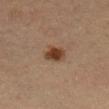Case summary:
• follow-up: catalogued during a skin exam; not biopsied
• tile lighting: cross-polarized illumination
• automated metrics: about 12 CIELAB-L* units darker than the surrounding skin and a normalized border contrast of about 10.5; a border-irregularity index near 1.5/10; a nevus-likeness score of about 100/100 and a detector confidence of about 100 out of 100 that the crop contains a lesion
• site: the right lower leg
• lesion diameter: ~2.5 mm (longest diameter)
• subject: female, about 30 years old
• image source: ~15 mm tile from a whole-body skin photo